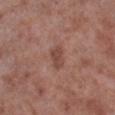workup = catalogued during a skin exam; not biopsied
image source = ~15 mm crop, total-body skin-cancer survey
subject = male, approximately 55 years of age
body site = the right lower leg
size = about 2.5 mm
tile lighting = white-light